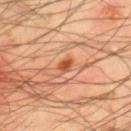Clinical summary:
A male patient, approximately 45 years of age. From the right lower leg. A 15 mm crop from a total-body photograph taken for skin-cancer surveillance. The recorded lesion diameter is about 2 mm. An algorithmic analysis of the crop reported a footprint of about 2 mm², a shape eccentricity near 0.65, and a shape-asymmetry score of about 0.3 (0 = symmetric). The analysis additionally found a border-irregularity index near 2.5/10. The analysis additionally found a classifier nevus-likeness of about 95/100 and lesion-presence confidence of about 100/100. Captured under cross-polarized illumination.